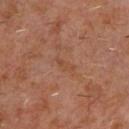Assessment: Captured during whole-body skin photography for melanoma surveillance; the lesion was not biopsied. Acquisition and patient details: A 15 mm crop from a total-body photograph taken for skin-cancer surveillance. Located on the front of the torso. The tile uses cross-polarized illumination. A male subject in their 60s. Approximately 2.5 mm at its widest.